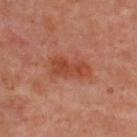Recorded during total-body skin imaging; not selected for excision or biopsy. The lesion-visualizer software estimated border irregularity of about 3 on a 0–10 scale, a within-lesion color-variation index near 3/10, and radial color variation of about 1. A male patient, aged around 50. A 15 mm crop from a total-body photograph taken for skin-cancer surveillance. The lesion is located on the upper back. Imaged with cross-polarized lighting. Approximately 5 mm at its widest.